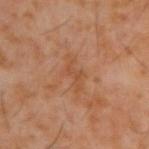Q: Was this lesion biopsied?
A: total-body-photography surveillance lesion; no biopsy
Q: Where on the body is the lesion?
A: the upper back
Q: Illumination type?
A: cross-polarized illumination
Q: What is the lesion's diameter?
A: ~3.5 mm (longest diameter)
Q: Automated lesion metrics?
A: a mean CIELAB color near L≈46 a*≈22 b*≈33, about 5 CIELAB-L* units darker than the surrounding skin, and a normalized border contrast of about 4.5
Q: How was this image acquired?
A: ~15 mm crop, total-body skin-cancer survey
Q: Patient demographics?
A: male, about 60 years old